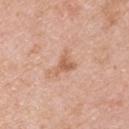A male subject, aged 48–52.
Cropped from a whole-body photographic skin survey; the tile spans about 15 mm.
This is a white-light tile.
The lesion is located on the right upper arm.
Automated tile analysis of the lesion measured a border-irregularity rating of about 5.5/10 and peripheral color asymmetry of about 1.
Approximately 3.5 mm at its widest.